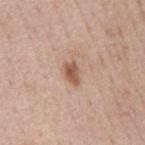The lesion was photographed on a routine skin check and not biopsied; there is no pathology result.
On the mid back.
The tile uses white-light illumination.
This image is a 15 mm lesion crop taken from a total-body photograph.
A male patient aged 48 to 52.
The lesion's longest dimension is about 3 mm.